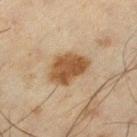Findings:
- workup · total-body-photography surveillance lesion; no biopsy